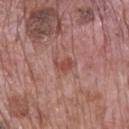From the mid back. A 15 mm close-up tile from a total-body photography series done for melanoma screening. A male patient, about 70 years old. The lesion's longest dimension is about 2.5 mm.